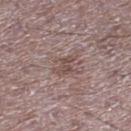From the right lower leg. Cropped from a whole-body photographic skin survey; the tile spans about 15 mm. Approximately 3.5 mm at its widest. The tile uses white-light illumination. A male subject, approximately 50 years of age. Automated tile analysis of the lesion measured a nevus-likeness score of about 0/100 and a lesion-detection confidence of about 75/100.